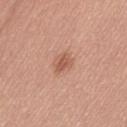Recorded during total-body skin imaging; not selected for excision or biopsy.
A male patient in their mid- to late 50s.
A 15 mm crop from a total-body photograph taken for skin-cancer surveillance.
Captured under white-light illumination.
From the left thigh.